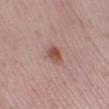{
  "biopsy_status": "not biopsied; imaged during a skin examination",
  "lighting": "white-light",
  "site": "left lower leg",
  "patient": {
    "sex": "female",
    "age_approx": 40
  },
  "image": {
    "source": "total-body photography crop",
    "field_of_view_mm": 15
  },
  "automated_metrics": {
    "cielab_L": 51,
    "cielab_a": 23,
    "cielab_b": 25,
    "vs_skin_darker_L": 11.0,
    "vs_skin_contrast_norm": 8.0,
    "border_irregularity_0_10": 1.5,
    "color_variation_0_10": 3.5
  }
}